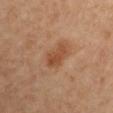Notes:
• follow-up · no biopsy performed (imaged during a skin exam)
• lesion size · about 3.5 mm
• patient · female, aged 43–47
• imaging modality · total-body-photography crop, ~15 mm field of view
• automated lesion analysis · an average lesion color of about L≈48 a*≈23 b*≈34 (CIELAB); border irregularity of about 2.5 on a 0–10 scale and a within-lesion color-variation index near 3/10
• anatomic site · the right upper arm
• lighting · cross-polarized